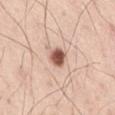| key | value |
|---|---|
| notes | total-body-photography surveillance lesion; no biopsy |
| image | ~15 mm crop, total-body skin-cancer survey |
| body site | the front of the torso |
| lesion size | ≈2.5 mm |
| subject | male, roughly 55 years of age |
| lighting | white-light illumination |
| automated lesion analysis | a mean CIELAB color near L≈56 a*≈22 b*≈28 and a lesion-to-skin contrast of about 11.5 (normalized; higher = more distinct) |